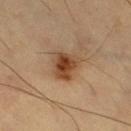| field | value |
|---|---|
| notes | no biopsy performed (imaged during a skin exam) |
| anatomic site | the right thigh |
| patient | male, aged approximately 60 |
| imaging modality | ~15 mm tile from a whole-body skin photo |
| illumination | cross-polarized illumination |